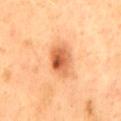workup = no biopsy performed (imaged during a skin exam) | anatomic site = the mid back | image = ~15 mm crop, total-body skin-cancer survey | illumination = cross-polarized illumination | subject = male, aged 48 to 52.